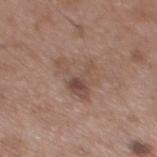Captured during whole-body skin photography for melanoma surveillance; the lesion was not biopsied.
A 15 mm crop from a total-body photograph taken for skin-cancer surveillance.
The lesion is on the back.
A male patient, about 55 years old.
Captured under white-light illumination.
About 5 mm across.
Automated tile analysis of the lesion measured a lesion area of about 9.5 mm², an outline eccentricity of about 0.6 (0 = round, 1 = elongated), and a symmetry-axis asymmetry near 0.65. It also reported a border-irregularity index near 9/10, a within-lesion color-variation index near 5.5/10, and a peripheral color-asymmetry measure near 1.5.